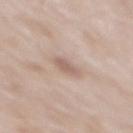Imaged during a routine full-body skin examination; the lesion was not biopsied and no histopathology is available. Imaged with white-light lighting. A male patient, aged 63–67. On the mid back. A roughly 15 mm field-of-view crop from a total-body skin photograph. The lesion's longest dimension is about 3 mm.